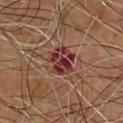<lesion>
  <biopsy_status>not biopsied; imaged during a skin examination</biopsy_status>
  <patient>
    <sex>male</sex>
    <age_approx>65</age_approx>
  </patient>
  <site>chest</site>
  <lesion_size>
    <long_diameter_mm_approx>3.5</long_diameter_mm_approx>
  </lesion_size>
  <automated_metrics>
    <area_mm2_approx>9.0</area_mm2_approx>
    <shape_asymmetry>0.25</shape_asymmetry>
    <lesion_detection_confidence_0_100>100</lesion_detection_confidence_0_100>
  </automated_metrics>
  <image>
    <source>total-body photography crop</source>
    <field_of_view_mm>15</field_of_view_mm>
  </image>
  <lighting>cross-polarized</lighting>
</lesion>